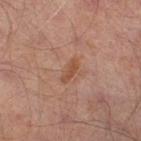Case summary:
* size — about 3 mm
* image-analysis metrics — a mean CIELAB color near L≈47 a*≈21 b*≈30, a lesion–skin lightness drop of about 7, and a normalized border contrast of about 6.5; a nevus-likeness score of about 5/100 and a detector confidence of about 100 out of 100 that the crop contains a lesion
* acquisition — ~15 mm crop, total-body skin-cancer survey
* subject — male, aged around 65
* illumination — cross-polarized
* site — the leg